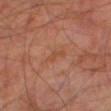follow-up: total-body-photography surveillance lesion; no biopsy